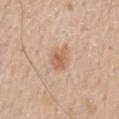Part of a total-body skin-imaging series; this lesion was reviewed on a skin check and was not flagged for biopsy. The lesion is on the back. Cropped from a total-body skin-imaging series; the visible field is about 15 mm. The patient is a male aged 58 to 62.